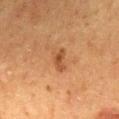Captured during whole-body skin photography for melanoma surveillance; the lesion was not biopsied. A 15 mm close-up extracted from a 3D total-body photography capture. The lesion is on the mid back. A male patient in their 60s. The total-body-photography lesion software estimated a footprint of about 4 mm². The analysis additionally found a lesion color around L≈46 a*≈22 b*≈36 in CIELAB and roughly 9 lightness units darker than nearby skin. Approximately 3 mm at its widest.